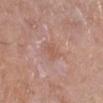Clinical summary:
Measured at roughly 2.5 mm in maximum diameter. A roughly 15 mm field-of-view crop from a total-body skin photograph. An algorithmic analysis of the crop reported a lesion area of about 5 mm², an outline eccentricity of about 0.5 (0 = round, 1 = elongated), and a shape-asymmetry score of about 0.3 (0 = symmetric). It also reported a detector confidence of about 100 out of 100 that the crop contains a lesion. The subject is a male roughly 70 years of age. On the left lower leg.